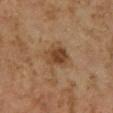Findings:
– follow-up: imaged on a skin check; not biopsied